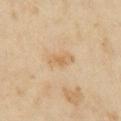<tbp_lesion>
<biopsy_status>not biopsied; imaged during a skin examination</biopsy_status>
<automated_metrics>
  <area_mm2_approx>5.5</area_mm2_approx>
  <eccentricity>0.85</eccentricity>
  <shape_asymmetry>0.25</shape_asymmetry>
  <color_variation_0_10>2.5</color_variation_0_10>
</automated_metrics>
<patient>
  <sex>female</sex>
  <age_approx>35</age_approx>
</patient>
<lighting>cross-polarized</lighting>
<image>
  <source>total-body photography crop</source>
  <field_of_view_mm>15</field_of_view_mm>
</image>
<site>right upper arm</site>
</tbp_lesion>